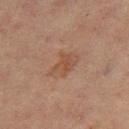Notes:
- notes · no biopsy performed (imaged during a skin exam)
- site · the leg
- image source · ~15 mm tile from a whole-body skin photo
- automated lesion analysis · an average lesion color of about L≈43 a*≈17 b*≈27 (CIELAB) and a normalized border contrast of about 5.5
- subject · female, aged approximately 55
- illumination · cross-polarized illumination
- diameter · ~4 mm (longest diameter)